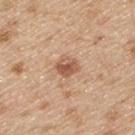Assessment:
No biopsy was performed on this lesion — it was imaged during a full skin examination and was not determined to be concerning.